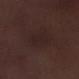Recorded during total-body skin imaging; not selected for excision or biopsy.
Automated tile analysis of the lesion measured an area of roughly 7.5 mm², an eccentricity of roughly 0.85, and a symmetry-axis asymmetry near 0.25. And it measured a mean CIELAB color near L≈21 a*≈14 b*≈14 and a lesion-to-skin contrast of about 4.5 (normalized; higher = more distinct).
A male subject approximately 70 years of age.
Captured under white-light illumination.
This image is a 15 mm lesion crop taken from a total-body photograph.
From the right lower leg.